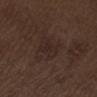biopsy status: catalogued during a skin exam; not biopsied | imaging modality: 15 mm crop, total-body photography | size: ~4 mm (longest diameter) | lighting: white-light | automated metrics: a footprint of about 7.5 mm², an eccentricity of roughly 0.85, and a shape-asymmetry score of about 0.3 (0 = symmetric); a lesion color around L≈24 a*≈14 b*≈17 in CIELAB and roughly 4 lightness units darker than nearby skin; a border-irregularity rating of about 3/10, internal color variation of about 2 on a 0–10 scale, and peripheral color asymmetry of about 1; a lesion-detection confidence of about 100/100 | anatomic site: the abdomen | patient: male, aged approximately 70.